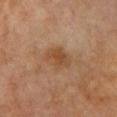notes: total-body-photography surveillance lesion; no biopsy
lighting: cross-polarized
size: about 3.5 mm
image: ~15 mm crop, total-body skin-cancer survey
site: the chest
automated metrics: a lesion area of about 7.5 mm² and two-axis asymmetry of about 0.2; a mean CIELAB color near L≈41 a*≈17 b*≈30, about 7 CIELAB-L* units darker than the surrounding skin, and a normalized border contrast of about 7; internal color variation of about 3 on a 0–10 scale and peripheral color asymmetry of about 1; a nevus-likeness score of about 0/100 and a lesion-detection confidence of about 100/100
subject: female, in their 70s